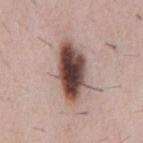Imaged during a routine full-body skin examination; the lesion was not biopsied and no histopathology is available. Approximately 7 mm at its widest. From the chest. Automated image analysis of the tile measured a border-irregularity rating of about 2.5/10 and radial color variation of about 2.5. The software also gave an automated nevus-likeness rating near 100 out of 100 and a detector confidence of about 100 out of 100 that the crop contains a lesion. A male patient, roughly 50 years of age. A close-up tile cropped from a whole-body skin photograph, about 15 mm across.